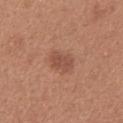Q: Was a biopsy performed?
A: no biopsy performed (imaged during a skin exam)
Q: Automated lesion metrics?
A: a lesion color around L≈50 a*≈23 b*≈29 in CIELAB, a lesion–skin lightness drop of about 8, and a lesion-to-skin contrast of about 6 (normalized; higher = more distinct); border irregularity of about 2.5 on a 0–10 scale and peripheral color asymmetry of about 0.5; a classifier nevus-likeness of about 45/100 and a lesion-detection confidence of about 100/100
Q: Illumination type?
A: white-light
Q: What is the imaging modality?
A: total-body-photography crop, ~15 mm field of view
Q: What is the lesion's diameter?
A: ~3 mm (longest diameter)
Q: Where on the body is the lesion?
A: the upper back
Q: Who is the patient?
A: female, roughly 40 years of age